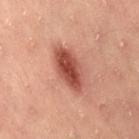Case summary:
– workup: imaged on a skin check; not biopsied
– image: 15 mm crop, total-body photography
– lesion size: ~5.5 mm (longest diameter)
– subject: female, roughly 35 years of age
– TBP lesion metrics: an area of roughly 11 mm²; a mean CIELAB color near L≈50 a*≈30 b*≈30, about 16 CIELAB-L* units darker than the surrounding skin, and a normalized lesion–skin contrast near 10.5; a border-irregularity rating of about 2.5/10, a within-lesion color-variation index near 4.5/10, and peripheral color asymmetry of about 1.5; a classifier nevus-likeness of about 95/100
– tile lighting: cross-polarized
– location: the back